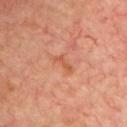• notes — total-body-photography surveillance lesion; no biopsy